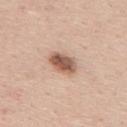notes: no biopsy performed (imaged during a skin exam)
image-analysis metrics: a footprint of about 7 mm², a shape eccentricity near 0.75, and a symmetry-axis asymmetry near 0.2; a mean CIELAB color near L≈57 a*≈20 b*≈29, a lesion–skin lightness drop of about 16, and a lesion-to-skin contrast of about 10 (normalized; higher = more distinct)
tile lighting: white-light illumination
anatomic site: the mid back
diameter: ~3.5 mm (longest diameter)
image source: ~15 mm crop, total-body skin-cancer survey
patient: male, approximately 45 years of age